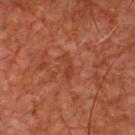No biopsy was performed on this lesion — it was imaged during a full skin examination and was not determined to be concerning. The lesion's longest dimension is about 3 mm. A region of skin cropped from a whole-body photographic capture, roughly 15 mm wide. On the chest. The subject is a male approximately 65 years of age. Imaged with cross-polarized lighting. An algorithmic analysis of the crop reported an outline eccentricity of about 0.9 (0 = round, 1 = elongated) and two-axis asymmetry of about 0.5. It also reported border irregularity of about 5 on a 0–10 scale, internal color variation of about 0 on a 0–10 scale, and radial color variation of about 0. And it measured a nevus-likeness score of about 0/100.